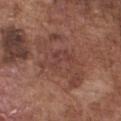Captured during whole-body skin photography for melanoma surveillance; the lesion was not biopsied.
On the chest.
A male subject roughly 75 years of age.
A 15 mm close-up tile from a total-body photography series done for melanoma screening.